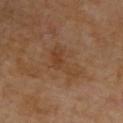<case>
  <biopsy_status>not biopsied; imaged during a skin examination</biopsy_status>
  <patient>
    <sex>male</sex>
    <age_approx>70</age_approx>
  </patient>
  <image>
    <source>total-body photography crop</source>
    <field_of_view_mm>15</field_of_view_mm>
  </image>
  <site>upper back</site>
</case>